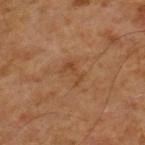Clinical impression:
No biopsy was performed on this lesion — it was imaged during a full skin examination and was not determined to be concerning.
Acquisition and patient details:
Approximately 3 mm at its widest. Imaged with cross-polarized lighting. A close-up tile cropped from a whole-body skin photograph, about 15 mm across. A male patient aged around 60. Located on the right lower leg. The lesion-visualizer software estimated an outline eccentricity of about 0.85 (0 = round, 1 = elongated) and a symmetry-axis asymmetry near 0.4. It also reported an average lesion color of about L≈42 a*≈20 b*≈33 (CIELAB), a lesion–skin lightness drop of about 6, and a normalized lesion–skin contrast near 5. The software also gave a lesion-detection confidence of about 100/100.